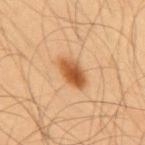| feature | finding |
|---|---|
| follow-up | catalogued during a skin exam; not biopsied |
| site | the mid back |
| patient | male, in their mid-50s |
| imaging modality | ~15 mm crop, total-body skin-cancer survey |